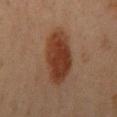Part of a total-body skin-imaging series; this lesion was reviewed on a skin check and was not flagged for biopsy. A female patient, roughly 45 years of age. The tile uses cross-polarized illumination. On the right upper arm. A close-up tile cropped from a whole-body skin photograph, about 15 mm across.